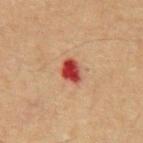{
  "biopsy_status": "not biopsied; imaged during a skin examination",
  "image": {
    "source": "total-body photography crop",
    "field_of_view_mm": 15
  },
  "lesion_size": {
    "long_diameter_mm_approx": 3.0
  },
  "lighting": "cross-polarized",
  "patient": {
    "sex": "male",
    "age_approx": 65
  },
  "site": "chest"
}